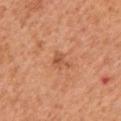TBP lesion metrics: a shape eccentricity near 0.75 and a shape-asymmetry score of about 0.55 (0 = symmetric); border irregularity of about 6 on a 0–10 scale, a color-variation rating of about 1.5/10, and peripheral color asymmetry of about 0.5; an automated nevus-likeness rating near 0 out of 100 and a detector confidence of about 100 out of 100 that the crop contains a lesion | location: the front of the torso | imaging modality: 15 mm crop, total-body photography | patient: male, approximately 55 years of age.